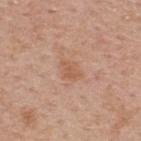Clinical impression: The lesion was tiled from a total-body skin photograph and was not biopsied. Acquisition and patient details: Automated tile analysis of the lesion measured an area of roughly 4.5 mm², a shape eccentricity near 0.7, and a shape-asymmetry score of about 0.35 (0 = symmetric). On the upper back. A 15 mm close-up extracted from a 3D total-body photography capture. Measured at roughly 3 mm in maximum diameter. A male patient, in their 60s. This is a white-light tile.